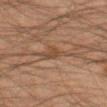Part of a total-body skin-imaging series; this lesion was reviewed on a skin check and was not flagged for biopsy. The lesion is on the left thigh. A male patient in their mid- to late 30s. Longest diameter approximately 3 mm. The lesion-visualizer software estimated a lesion area of about 4.5 mm² and a shape-asymmetry score of about 0.35 (0 = symmetric). It also reported about 6 CIELAB-L* units darker than the surrounding skin and a normalized border contrast of about 5.5. The tile uses cross-polarized illumination. Cropped from a total-body skin-imaging series; the visible field is about 15 mm.